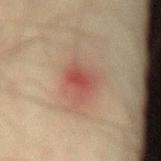The lesion was tiled from a total-body skin photograph and was not biopsied.
Located on the mid back.
The patient is a male approximately 75 years of age.
Approximately 3.5 mm at its widest.
A close-up tile cropped from a whole-body skin photograph, about 15 mm across.